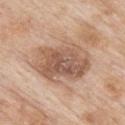{
  "biopsy_status": "not biopsied; imaged during a skin examination",
  "site": "upper back",
  "patient": {
    "sex": "male",
    "age_approx": "85+"
  },
  "image": {
    "source": "total-body photography crop",
    "field_of_view_mm": 15
  },
  "lesion_size": {
    "long_diameter_mm_approx": 7.5
  }
}